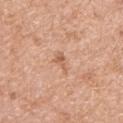Q: Is there a histopathology result?
A: imaged on a skin check; not biopsied
Q: What is the lesion's diameter?
A: ~2.5 mm (longest diameter)
Q: What lighting was used for the tile?
A: white-light
Q: Lesion location?
A: the right upper arm
Q: What are the patient's age and sex?
A: female, aged around 70
Q: How was this image acquired?
A: ~15 mm tile from a whole-body skin photo